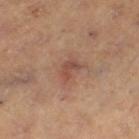{"biopsy_status": "not biopsied; imaged during a skin examination", "lesion_size": {"long_diameter_mm_approx": 2.5}, "site": "left thigh", "image": {"source": "total-body photography crop", "field_of_view_mm": 15}, "automated_metrics": {"nevus_likeness_0_100": 10, "lesion_detection_confidence_0_100": 100}, "lighting": "cross-polarized", "patient": {"sex": "female", "age_approx": 40}}